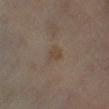| field | value |
|---|---|
| follow-up | imaged on a skin check; not biopsied |
| acquisition | ~15 mm crop, total-body skin-cancer survey |
| patient | female, aged 58–62 |
| lesion diameter | ≈3 mm |
| lighting | cross-polarized illumination |
| body site | the leg |